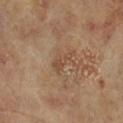| feature | finding |
|---|---|
| biopsy status | imaged on a skin check; not biopsied |
| subject | female, aged 73 to 77 |
| image | ~15 mm crop, total-body skin-cancer survey |
| tile lighting | cross-polarized illumination |
| diameter | ≈3 mm |
| body site | the leg |